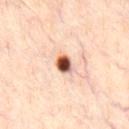Impression: Imaged during a routine full-body skin examination; the lesion was not biopsied and no histopathology is available. Image and clinical context: Approximately 2.5 mm at its widest. Imaged with cross-polarized lighting. The lesion is on the chest. A close-up tile cropped from a whole-body skin photograph, about 15 mm across. A male subject, in their mid-30s.